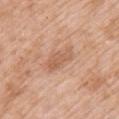notes = total-body-photography surveillance lesion; no biopsy
anatomic site = the left upper arm
illumination = white-light illumination
patient = female, in their 70s
lesion size = ≈3 mm
image source = ~15 mm tile from a whole-body skin photo